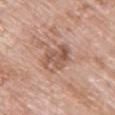Clinical impression:
Captured during whole-body skin photography for melanoma surveillance; the lesion was not biopsied.
Acquisition and patient details:
The patient is a female approximately 85 years of age or older. This is a white-light tile. A 15 mm close-up tile from a total-body photography series done for melanoma screening. Located on the upper back. About 4 mm across.